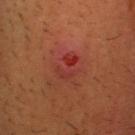Assessment: Part of a total-body skin-imaging series; this lesion was reviewed on a skin check and was not flagged for biopsy. Background: A roughly 15 mm field-of-view crop from a total-body skin photograph. Automated tile analysis of the lesion measured a lesion color around L≈35 a*≈33 b*≈30 in CIELAB and a normalized lesion–skin contrast near 5.5. About 3 mm across. This is a cross-polarized tile. A male patient. The lesion is on the head or neck.